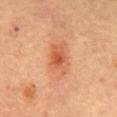Clinical impression:
The lesion was tiled from a total-body skin photograph and was not biopsied.
Clinical summary:
This image is a 15 mm lesion crop taken from a total-body photograph. Located on the abdomen. Imaged with cross-polarized lighting. A female subject about 60 years old. The lesion-visualizer software estimated an average lesion color of about L≈51 a*≈26 b*≈35 (CIELAB) and a normalized lesion–skin contrast near 7. Longest diameter approximately 4 mm.